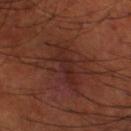workup = imaged on a skin check; not biopsied
patient = male, aged 68–72
imaging modality = ~15 mm crop, total-body skin-cancer survey
size = ~7 mm (longest diameter)
illumination = cross-polarized illumination
body site = the left lower leg